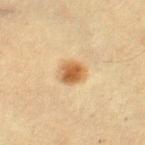The lesion was photographed on a routine skin check and not biopsied; there is no pathology result.
The patient is a female in their mid-50s.
The lesion is on the right thigh.
This is a cross-polarized tile.
A 15 mm close-up tile from a total-body photography series done for melanoma screening.